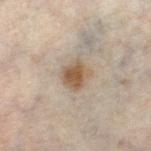Q: Patient demographics?
A: female, aged 48–52
Q: Lesion location?
A: the left thigh
Q: What kind of image is this?
A: 15 mm crop, total-body photography